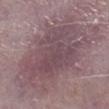The lesion was photographed on a routine skin check and not biopsied; there is no pathology result. Imaged with white-light lighting. A lesion tile, about 15 mm wide, cut from a 3D total-body photograph. The lesion is on the right lower leg. A male subject aged 73–77.